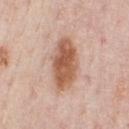The lesion was photographed on a routine skin check and not biopsied; there is no pathology result. The subject is a male approximately 60 years of age. A roughly 15 mm field-of-view crop from a total-body skin photograph. Approximately 6.5 mm at its widest. Located on the chest. Automated image analysis of the tile measured an outline eccentricity of about 0.85 (0 = round, 1 = elongated) and a symmetry-axis asymmetry near 0.15. The analysis additionally found an average lesion color of about L≈58 a*≈22 b*≈31 (CIELAB), about 14 CIELAB-L* units darker than the surrounding skin, and a lesion-to-skin contrast of about 10 (normalized; higher = more distinct). The analysis additionally found border irregularity of about 2 on a 0–10 scale and a peripheral color-asymmetry measure near 1.5. Captured under white-light illumination.